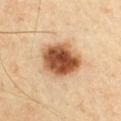imaging modality: ~15 mm crop, total-body skin-cancer survey | location: the chest | TBP lesion metrics: a shape eccentricity near 0.55 and two-axis asymmetry of about 0.15; an average lesion color of about L≈48 a*≈21 b*≈32 (CIELAB), about 19 CIELAB-L* units darker than the surrounding skin, and a lesion-to-skin contrast of about 12.5 (normalized; higher = more distinct); a classifier nevus-likeness of about 100/100 | patient: male, about 40 years old | diameter: ≈5 mm | tile lighting: cross-polarized illumination.